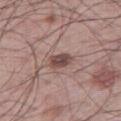Clinical impression: The lesion was photographed on a routine skin check and not biopsied; there is no pathology result. Clinical summary: The patient is a male roughly 60 years of age. The total-body-photography lesion software estimated an automated nevus-likeness rating near 80 out of 100. The lesion is on the right thigh. A 15 mm crop from a total-body photograph taken for skin-cancer surveillance.